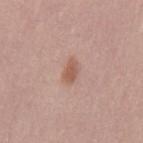Part of a total-body skin-imaging series; this lesion was reviewed on a skin check and was not flagged for biopsy. This is a white-light tile. The recorded lesion diameter is about 3 mm. A male patient, aged around 30. An algorithmic analysis of the crop reported an average lesion color of about L≈57 a*≈21 b*≈27 (CIELAB), a lesion–skin lightness drop of about 9, and a normalized border contrast of about 7. It also reported lesion-presence confidence of about 100/100. A 15 mm crop from a total-body photograph taken for skin-cancer surveillance.